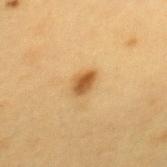{
  "biopsy_status": "not biopsied; imaged during a skin examination",
  "patient": {
    "sex": "female",
    "age_approx": 30
  },
  "site": "upper back",
  "image": {
    "source": "total-body photography crop",
    "field_of_view_mm": 15
  }
}